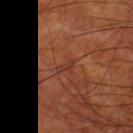<record>
  <biopsy_status>not biopsied; imaged during a skin examination</biopsy_status>
  <lighting>cross-polarized</lighting>
  <lesion_size>
    <long_diameter_mm_approx>2.5</long_diameter_mm_approx>
  </lesion_size>
  <site>left thigh</site>
  <image>
    <source>total-body photography crop</source>
    <field_of_view_mm>15</field_of_view_mm>
  </image>
  <automated_metrics>
    <eccentricity>0.9</eccentricity>
    <shape_asymmetry>0.4</shape_asymmetry>
    <cielab_L>35</cielab_L>
    <cielab_a>25</cielab_a>
    <cielab_b>29</cielab_b>
    <vs_skin_darker_L>5.0</vs_skin_darker_L>
    <vs_skin_contrast_norm>5.0</vs_skin_contrast_norm>
    <border_irregularity_0_10>4.5</border_irregularity_0_10>
    <color_variation_0_10>0.0</color_variation_0_10>
    <peripheral_color_asymmetry>0.0</peripheral_color_asymmetry>
    <nevus_likeness_0_100>0</nevus_likeness_0_100>
  </automated_metrics>
  <patient>
    <sex>male</sex>
    <age_approx>70</age_approx>
  </patient>
</record>